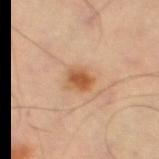Q: Was a biopsy performed?
A: imaged on a skin check; not biopsied
Q: Automated lesion metrics?
A: an area of roughly 5 mm² and a shape-asymmetry score of about 0.25 (0 = symmetric); a lesion–skin lightness drop of about 12 and a lesion-to-skin contrast of about 9 (normalized; higher = more distinct)
Q: What is the imaging modality?
A: total-body-photography crop, ~15 mm field of view
Q: What lighting was used for the tile?
A: cross-polarized
Q: Lesion location?
A: the left thigh
Q: Lesion size?
A: ≈2.5 mm